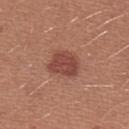Q: Is there a histopathology result?
A: imaged on a skin check; not biopsied
Q: What is the imaging modality?
A: ~15 mm crop, total-body skin-cancer survey
Q: Patient demographics?
A: female, approximately 25 years of age
Q: Lesion location?
A: the arm